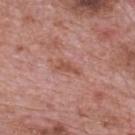Case summary:
• biopsy status: imaged on a skin check; not biopsied
• image-analysis metrics: an area of roughly 3.5 mm², an outline eccentricity of about 0.9 (0 = round, 1 = elongated), and a shape-asymmetry score of about 0.5 (0 = symmetric); a lesion color around L≈52 a*≈24 b*≈28 in CIELAB and a normalized lesion–skin contrast near 6.5; a border-irregularity index near 5.5/10, a within-lesion color-variation index near 0/10, and radial color variation of about 0; a classifier nevus-likeness of about 0/100 and a detector confidence of about 100 out of 100 that the crop contains a lesion
• subject: male, approximately 70 years of age
• diameter: ≈3 mm
• tile lighting: white-light illumination
• body site: the mid back
• acquisition: ~15 mm tile from a whole-body skin photo